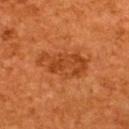notes = imaged on a skin check; not biopsied | illumination = cross-polarized illumination | body site = the upper back | lesion size = ~6 mm (longest diameter) | image source = ~15 mm crop, total-body skin-cancer survey | patient = male, about 45 years old | TBP lesion metrics = a border-irregularity index near 3/10, internal color variation of about 4 on a 0–10 scale, and radial color variation of about 1.5.